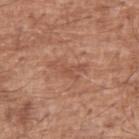{
  "biopsy_status": "not biopsied; imaged during a skin examination",
  "site": "left upper arm",
  "image": {
    "source": "total-body photography crop",
    "field_of_view_mm": 15
  },
  "patient": {
    "sex": "male",
    "age_approx": 65
  }
}